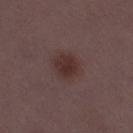Image and clinical context:
Located on the leg. This is a white-light tile. Cropped from a whole-body photographic skin survey; the tile spans about 15 mm. The recorded lesion diameter is about 3 mm. The patient is a female aged approximately 30.